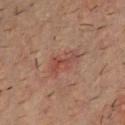The lesion was tiled from a total-body skin photograph and was not biopsied. Cropped from a total-body skin-imaging series; the visible field is about 15 mm. Imaged with cross-polarized lighting. On the chest. The lesion's longest dimension is about 4 mm. A male subject in their mid-30s.